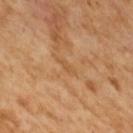{"biopsy_status": "not biopsied; imaged during a skin examination", "patient": {"sex": "male", "age_approx": 65}, "image": {"source": "total-body photography crop", "field_of_view_mm": 15}, "lighting": "cross-polarized", "automated_metrics": {"border_irregularity_0_10": 6.5, "peripheral_color_asymmetry": 0.0}}